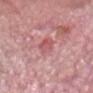Clinical impression:
Part of a total-body skin-imaging series; this lesion was reviewed on a skin check and was not flagged for biopsy.
Acquisition and patient details:
The lesion is located on the head or neck. A male patient, aged 58 to 62. A roughly 15 mm field-of-view crop from a total-body skin photograph.